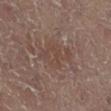Q: Is there a histopathology result?
A: imaged on a skin check; not biopsied
Q: What lighting was used for the tile?
A: cross-polarized illumination
Q: How large is the lesion?
A: ≈4.5 mm
Q: What is the anatomic site?
A: the right leg
Q: What are the patient's age and sex?
A: female, about 80 years old
Q: What is the imaging modality?
A: total-body-photography crop, ~15 mm field of view
Q: Automated lesion metrics?
A: an area of roughly 10 mm², an outline eccentricity of about 0.7 (0 = round, 1 = elongated), and a shape-asymmetry score of about 0.3 (0 = symmetric); a lesion color around L≈38 a*≈15 b*≈21 in CIELAB and a normalized lesion–skin contrast near 4.5; a border-irregularity index near 4.5/10, a within-lesion color-variation index near 2/10, and peripheral color asymmetry of about 0.5; an automated nevus-likeness rating near 0 out of 100 and lesion-presence confidence of about 95/100